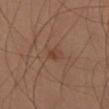This image is a 15 mm lesion crop taken from a total-body photograph.
The lesion is on the right thigh.
A male subject aged approximately 55.
Captured under cross-polarized illumination.
About 2 mm across.